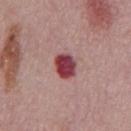biopsy_status: not biopsied; imaged during a skin examination
site: abdomen
image:
  source: total-body photography crop
  field_of_view_mm: 15
patient:
  sex: male
  age_approx: 75